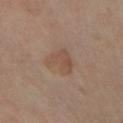Captured during whole-body skin photography for melanoma surveillance; the lesion was not biopsied. The lesion is located on the left lower leg. This is a cross-polarized tile. The subject is a female in their 50s. Cropped from a whole-body photographic skin survey; the tile spans about 15 mm.